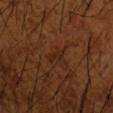Impression: The lesion was tiled from a total-body skin photograph and was not biopsied. Context: A roughly 15 mm field-of-view crop from a total-body skin photograph. Captured under cross-polarized illumination. A male subject, roughly 65 years of age. The lesion is on the right forearm.